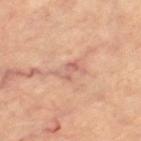biopsy status: total-body-photography surveillance lesion; no biopsy
body site: the left thigh
image-analysis metrics: a lesion color around L≈59 a*≈22 b*≈27 in CIELAB, a lesion–skin lightness drop of about 7, and a normalized lesion–skin contrast near 5.5; a classifier nevus-likeness of about 0/100
imaging modality: ~15 mm crop, total-body skin-cancer survey
lesion size: ≈3 mm
patient: female, about 65 years old